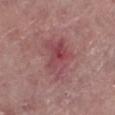This lesion was catalogued during total-body skin photography and was not selected for biopsy.
The total-body-photography lesion software estimated a footprint of about 15 mm² and a symmetry-axis asymmetry near 0.35. The software also gave about 8 CIELAB-L* units darker than the surrounding skin. And it measured a classifier nevus-likeness of about 0/100 and a lesion-detection confidence of about 100/100.
The patient is a female about 60 years old.
The recorded lesion diameter is about 5.5 mm.
Imaged with white-light lighting.
The lesion is located on the left lower leg.
A 15 mm close-up tile from a total-body photography series done for melanoma screening.